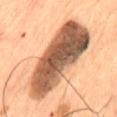workup=no biopsy performed (imaged during a skin exam)
subject=male, in their mid- to late 50s
anatomic site=the abdomen
acquisition=~15 mm crop, total-body skin-cancer survey
lighting=cross-polarized
diameter=about 11 mm